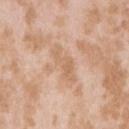Clinical impression:
Imaged during a routine full-body skin examination; the lesion was not biopsied and no histopathology is available.
Context:
From the right upper arm. A 15 mm close-up tile from a total-body photography series done for melanoma screening. Longest diameter approximately 4.5 mm. Imaged with white-light lighting. An algorithmic analysis of the crop reported a footprint of about 6.5 mm², a shape eccentricity near 0.9, and a shape-asymmetry score of about 0.45 (0 = symmetric). It also reported a lesion color around L≈64 a*≈19 b*≈33 in CIELAB, about 7 CIELAB-L* units darker than the surrounding skin, and a normalized border contrast of about 5. The analysis additionally found border irregularity of about 6 on a 0–10 scale. And it measured a nevus-likeness score of about 0/100 and a detector confidence of about 95 out of 100 that the crop contains a lesion. A female patient, in their mid- to late 20s.